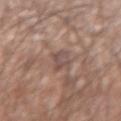Context:
An algorithmic analysis of the crop reported an area of roughly 4.5 mm², an eccentricity of roughly 0.85, and a symmetry-axis asymmetry near 0.4. It also reported a classifier nevus-likeness of about 0/100 and a detector confidence of about 60 out of 100 that the crop contains a lesion. The lesion's longest dimension is about 3.5 mm. The tile uses white-light illumination. Located on the left upper arm. A roughly 15 mm field-of-view crop from a total-body skin photograph. A male subject, aged 63–67.